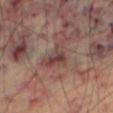- notes: imaged on a skin check; not biopsied
- patient: male, aged 68 to 72
- tile lighting: cross-polarized illumination
- imaging modality: 15 mm crop, total-body photography
- diameter: ≈5 mm
- site: the right thigh